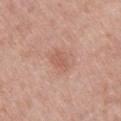Q: Was a biopsy performed?
A: total-body-photography surveillance lesion; no biopsy
Q: Who is the patient?
A: male, approximately 75 years of age
Q: Lesion location?
A: the right thigh
Q: How was this image acquired?
A: total-body-photography crop, ~15 mm field of view